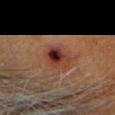The lesion was tiled from a total-body skin photograph and was not biopsied.